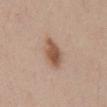biopsy_status: not biopsied; imaged during a skin examination
patient:
  sex: male
  age_approx: 40
image:
  source: total-body photography crop
  field_of_view_mm: 15
site: front of the torso